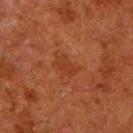| field | value |
|---|---|
| workup | no biopsy performed (imaged during a skin exam) |
| image | 15 mm crop, total-body photography |
| anatomic site | the right lower leg |
| diameter | ≈3 mm |
| subject | male, in their 80s |
| lighting | cross-polarized illumination |